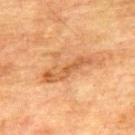A male patient aged 73 to 77. The total-body-photography lesion software estimated a lesion area of about 12 mm², a shape eccentricity near 0.9, and two-axis asymmetry of about 0.5. The analysis additionally found a lesion color around L≈50 a*≈21 b*≈36 in CIELAB. It also reported border irregularity of about 8 on a 0–10 scale, a within-lesion color-variation index near 4.5/10, and a peripheral color-asymmetry measure near 1.5. The analysis additionally found an automated nevus-likeness rating near 0 out of 100. Located on the upper back. This image is a 15 mm lesion crop taken from a total-body photograph. Imaged with cross-polarized lighting. About 7 mm across.